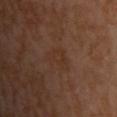subject = male, approximately 55 years of age | anatomic site = the front of the torso | lesion size = about 3.5 mm | illumination = cross-polarized illumination | imaging modality = 15 mm crop, total-body photography.